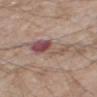Impression:
Captured during whole-body skin photography for melanoma surveillance; the lesion was not biopsied.
Context:
A lesion tile, about 15 mm wide, cut from a 3D total-body photograph. An algorithmic analysis of the crop reported an area of roughly 11 mm², a shape eccentricity near 0.9, and a shape-asymmetry score of about 0.3 (0 = symmetric). The analysis additionally found a border-irregularity index near 4.5/10 and a color-variation rating of about 10/10. A male subject aged approximately 70. On the right thigh. Longest diameter approximately 5.5 mm. Captured under white-light illumination.